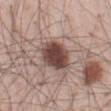The lesion was photographed on a routine skin check and not biopsied; there is no pathology result.
A 15 mm close-up tile from a total-body photography series done for melanoma screening.
A male subject aged around 55.
On the front of the torso.
Automated image analysis of the tile measured a border-irregularity index near 2/10, a color-variation rating of about 4.5/10, and radial color variation of about 1. And it measured an automated nevus-likeness rating near 95 out of 100 and a lesion-detection confidence of about 100/100.
The tile uses white-light illumination.